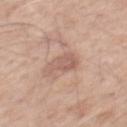Notes:
* workup · total-body-photography surveillance lesion; no biopsy
* acquisition · ~15 mm tile from a whole-body skin photo
* location · the mid back
* subject · male, roughly 60 years of age